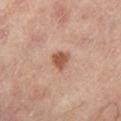biopsy status: total-body-photography surveillance lesion; no biopsy
image: 15 mm crop, total-body photography
automated lesion analysis: a lesion area of about 4 mm², an eccentricity of roughly 0.35, and two-axis asymmetry of about 0.25; a lesion color around L≈51 a*≈23 b*≈30 in CIELAB, about 12 CIELAB-L* units darker than the surrounding skin, and a normalized border contrast of about 9; a nevus-likeness score of about 90/100 and a lesion-detection confidence of about 100/100
site: the left lower leg
patient: male, aged around 65
lesion size: about 2.5 mm
lighting: cross-polarized illumination